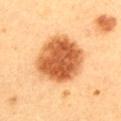workup — no biopsy performed (imaged during a skin exam); lesion size — ~6.5 mm (longest diameter); subject — female, about 30 years old; anatomic site — the back; image — 15 mm crop, total-body photography.